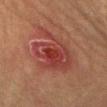Q: What is the anatomic site?
A: the abdomen
Q: Patient demographics?
A: female, aged 58 to 62
Q: How large is the lesion?
A: about 7.5 mm
Q: What is the imaging modality?
A: ~15 mm crop, total-body skin-cancer survey
Q: What lighting was used for the tile?
A: cross-polarized illumination